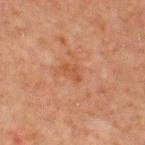notes: total-body-photography surveillance lesion; no biopsy
anatomic site: the front of the torso
subject: male, aged around 65
lesion size: ≈2.5 mm
image source: ~15 mm crop, total-body skin-cancer survey
tile lighting: cross-polarized